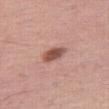No biopsy was performed on this lesion — it was imaged during a full skin examination and was not determined to be concerning. A female patient, roughly 50 years of age. Automated tile analysis of the lesion measured an area of roughly 5 mm², an outline eccentricity of about 0.75 (0 = round, 1 = elongated), and two-axis asymmetry of about 0.25. It also reported an average lesion color of about L≈52 a*≈24 b*≈25 (CIELAB), about 14 CIELAB-L* units darker than the surrounding skin, and a normalized border contrast of about 9. The software also gave an automated nevus-likeness rating near 90 out of 100 and a detector confidence of about 100 out of 100 that the crop contains a lesion. A 15 mm close-up extracted from a 3D total-body photography capture. From the right thigh.